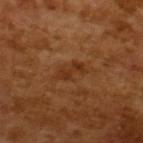The lesion was photographed on a routine skin check and not biopsied; there is no pathology result.
Approximately 3.5 mm at its widest.
A 15 mm close-up extracted from a 3D total-body photography capture.
Imaged with cross-polarized lighting.
The patient is a male aged 63 to 67.
Automated tile analysis of the lesion measured a lesion area of about 4 mm² and a symmetry-axis asymmetry near 0.45. The analysis additionally found a mean CIELAB color near L≈32 a*≈23 b*≈34, about 7 CIELAB-L* units darker than the surrounding skin, and a normalized lesion–skin contrast near 6.5. And it measured lesion-presence confidence of about 100/100.